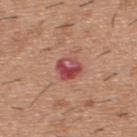notes = imaged on a skin check; not biopsied | imaging modality = ~15 mm tile from a whole-body skin photo | patient = male, approximately 40 years of age | lesion diameter = about 2.5 mm | location = the upper back.